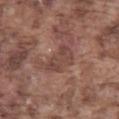biopsy_status: not biopsied; imaged during a skin examination
lighting: white-light
site: left thigh
lesion_size:
  long_diameter_mm_approx: 4.5
image:
  source: total-body photography crop
  field_of_view_mm: 15
patient:
  sex: male
  age_approx: 75